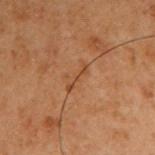follow-up = imaged on a skin check; not biopsied
subject = male, aged 58 to 62
lesion size = about 3.5 mm
imaging modality = total-body-photography crop, ~15 mm field of view
illumination = cross-polarized
site = the right upper arm